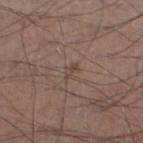Findings:
- biopsy status: no biopsy performed (imaged during a skin exam)
- TBP lesion metrics: an eccentricity of roughly 0.8 and two-axis asymmetry of about 0.35; border irregularity of about 4 on a 0–10 scale, a within-lesion color-variation index near 1/10, and a peripheral color-asymmetry measure near 0; a nevus-likeness score of about 0/100 and a detector confidence of about 90 out of 100 that the crop contains a lesion
- anatomic site: the left lower leg
- imaging modality: ~15 mm tile from a whole-body skin photo
- subject: male, aged 48 to 52
- lesion size: about 2.5 mm
- lighting: white-light